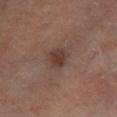A male subject aged approximately 65. About 2.5 mm across. Imaged with cross-polarized lighting. The lesion is on the right lower leg. A 15 mm crop from a total-body photograph taken for skin-cancer surveillance.